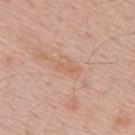Impression: The lesion was tiled from a total-body skin photograph and was not biopsied. Context: The tile uses white-light illumination. A region of skin cropped from a whole-body photographic capture, roughly 15 mm wide. A male patient, aged 53–57. The total-body-photography lesion software estimated a footprint of about 4 mm² and an outline eccentricity of about 0.9 (0 = round, 1 = elongated). The analysis additionally found a mean CIELAB color near L≈62 a*≈20 b*≈32, roughly 6 lightness units darker than nearby skin, and a lesion-to-skin contrast of about 5 (normalized; higher = more distinct). And it measured a border-irregularity rating of about 3.5/10, a within-lesion color-variation index near 1/10, and radial color variation of about 0. On the upper back. The lesion's longest dimension is about 3.5 mm.